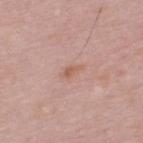Case summary:
* biopsy status · no biopsy performed (imaged during a skin exam)
* anatomic site · the upper back
* automated metrics · a footprint of about 2.5 mm², an eccentricity of roughly 0.9, and a shape-asymmetry score of about 0.35 (0 = symmetric); a lesion color around L≈59 a*≈22 b*≈29 in CIELAB, roughly 7 lightness units darker than nearby skin, and a normalized lesion–skin contrast near 6.5; a nevus-likeness score of about 0/100 and a lesion-detection confidence of about 100/100
* subject · male, in their 50s
* image · ~15 mm crop, total-body skin-cancer survey